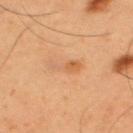workup=imaged on a skin check; not biopsied | anatomic site=the upper back | patient=male, about 55 years old | image=15 mm crop, total-body photography.